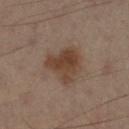The lesion was tiled from a total-body skin photograph and was not biopsied.
Automated tile analysis of the lesion measured a lesion area of about 12 mm² and two-axis asymmetry of about 0.35. The analysis additionally found a mean CIELAB color near L≈32 a*≈13 b*≈22 and a lesion-to-skin contrast of about 8.5 (normalized; higher = more distinct). The software also gave a nevus-likeness score of about 65/100 and a detector confidence of about 100 out of 100 that the crop contains a lesion.
A male subject, aged around 55.
Approximately 4.5 mm at its widest.
The lesion is on the left lower leg.
A close-up tile cropped from a whole-body skin photograph, about 15 mm across.